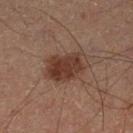workup = total-body-photography surveillance lesion; no biopsy
anatomic site = the left lower leg
image source = total-body-photography crop, ~15 mm field of view
lesion diameter = about 5.5 mm
subject = male, aged 48 to 52